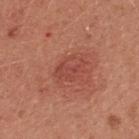Case summary:
* workup · no biopsy performed (imaged during a skin exam)
* diameter · ~3.5 mm (longest diameter)
* lighting · white-light illumination
* anatomic site · the chest
* image · ~15 mm tile from a whole-body skin photo
* patient · female, aged around 35
* image-analysis metrics · a border-irregularity rating of about 2/10, a color-variation rating of about 3/10, and radial color variation of about 1